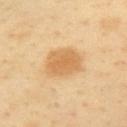Impression:
Imaged during a routine full-body skin examination; the lesion was not biopsied and no histopathology is available.
Acquisition and patient details:
A 15 mm close-up tile from a total-body photography series done for melanoma screening. The lesion is on the left upper arm. The patient is a male roughly 40 years of age.